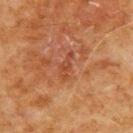| feature | finding |
|---|---|
| acquisition | ~15 mm crop, total-body skin-cancer survey |
| lighting | cross-polarized illumination |
| site | the back |
| subject | male, aged 78 to 82 |